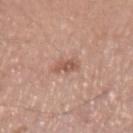Measured at roughly 2.5 mm in maximum diameter.
Automated image analysis of the tile measured an area of roughly 4 mm², a shape eccentricity near 0.75, and a symmetry-axis asymmetry near 0.3. And it measured a within-lesion color-variation index near 2.5/10 and radial color variation of about 1. The analysis additionally found lesion-presence confidence of about 100/100.
A male subject, aged 53 to 57.
The lesion is located on the left upper arm.
Imaged with white-light lighting.
A close-up tile cropped from a whole-body skin photograph, about 15 mm across.